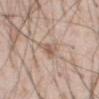Clinical impression: Imaged during a routine full-body skin examination; the lesion was not biopsied and no histopathology is available. Context: The lesion-visualizer software estimated an eccentricity of roughly 0.55. The analysis additionally found a border-irregularity index near 5.5/10, internal color variation of about 3.5 on a 0–10 scale, and a peripheral color-asymmetry measure near 1. Longest diameter approximately 3.5 mm. The lesion is located on the abdomen. This image is a 15 mm lesion crop taken from a total-body photograph. The tile uses white-light illumination. A male subject approximately 55 years of age.